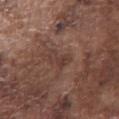• follow-up: total-body-photography surveillance lesion; no biopsy
• subject: male, in their mid- to late 70s
• image-analysis metrics: a border-irregularity index near 4.5/10, a within-lesion color-variation index near 1.5/10, and a peripheral color-asymmetry measure near 0.5
• illumination: white-light
• image: 15 mm crop, total-body photography
• anatomic site: the front of the torso